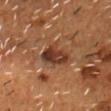{"biopsy_status": "not biopsied; imaged during a skin examination", "image": {"source": "total-body photography crop", "field_of_view_mm": 15}, "patient": {"sex": "male", "age_approx": 55}, "lesion_size": {"long_diameter_mm_approx": 3.5}, "lighting": "cross-polarized", "site": "head or neck"}